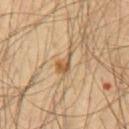Recorded during total-body skin imaging; not selected for excision or biopsy. This image is a 15 mm lesion crop taken from a total-body photograph. The subject is a male roughly 55 years of age. From the chest.